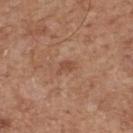The lesion was photographed on a routine skin check and not biopsied; there is no pathology result. Measured at roughly 2.5 mm in maximum diameter. The tile uses white-light illumination. From the upper back. A male subject aged approximately 65. Cropped from a total-body skin-imaging series; the visible field is about 15 mm. The total-body-photography lesion software estimated an area of roughly 2.5 mm², an outline eccentricity of about 0.85 (0 = round, 1 = elongated), and a symmetry-axis asymmetry near 0.4. The analysis additionally found internal color variation of about 0.5 on a 0–10 scale and radial color variation of about 0.